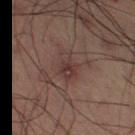Impression: Part of a total-body skin-imaging series; this lesion was reviewed on a skin check and was not flagged for biopsy.